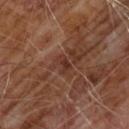Q: Is there a histopathology result?
A: no biopsy performed (imaged during a skin exam)
Q: What are the patient's age and sex?
A: male, aged 58–62
Q: What did automated image analysis measure?
A: an automated nevus-likeness rating near 0 out of 100 and a detector confidence of about 95 out of 100 that the crop contains a lesion
Q: How was this image acquired?
A: 15 mm crop, total-body photography
Q: How was the tile lit?
A: cross-polarized
Q: What is the anatomic site?
A: the chest